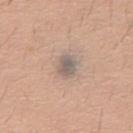Case summary:
– notes — catalogued during a skin exam; not biopsied
– patient — male, aged 58–62
– diameter — ≈3 mm
– automated lesion analysis — a lesion color around L≈58 a*≈11 b*≈21 in CIELAB, about 10 CIELAB-L* units darker than the surrounding skin, and a lesion-to-skin contrast of about 8 (normalized; higher = more distinct); border irregularity of about 1.5 on a 0–10 scale, a within-lesion color-variation index near 3.5/10, and radial color variation of about 1
– acquisition — total-body-photography crop, ~15 mm field of view
– illumination — white-light illumination
– site — the front of the torso